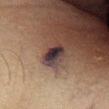follow-up=no biopsy performed (imaged during a skin exam)
diameter=~3 mm (longest diameter)
tile lighting=cross-polarized
patient=male, aged around 45
anatomic site=the right lower leg
imaging modality=total-body-photography crop, ~15 mm field of view